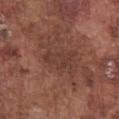The lesion was photographed on a routine skin check and not biopsied; there is no pathology result. The subject is a male approximately 75 years of age. Cropped from a total-body skin-imaging series; the visible field is about 15 mm. The lesion is on the chest. Imaged with white-light lighting. Measured at roughly 4 mm in maximum diameter.